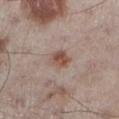The lesion was photographed on a routine skin check and not biopsied; there is no pathology result.